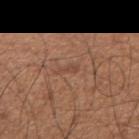Assessment:
Imaged during a routine full-body skin examination; the lesion was not biopsied and no histopathology is available.
Background:
Imaged with white-light lighting. The subject is a male approximately 65 years of age. A 15 mm close-up tile from a total-body photography series done for melanoma screening. The recorded lesion diameter is about 2.5 mm. Located on the arm. The lesion-visualizer software estimated a lesion area of about 2 mm². It also reported a border-irregularity rating of about 4/10, internal color variation of about 0 on a 0–10 scale, and peripheral color asymmetry of about 0.